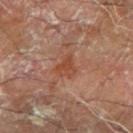Impression:
Captured during whole-body skin photography for melanoma surveillance; the lesion was not biopsied.
Background:
The subject is a male in their mid-60s. Cropped from a whole-body photographic skin survey; the tile spans about 15 mm. On the right forearm.